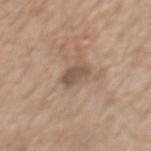Captured during whole-body skin photography for melanoma surveillance; the lesion was not biopsied.
The lesion is on the chest.
A 15 mm close-up extracted from a 3D total-body photography capture.
The subject is a male aged around 70.